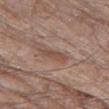Q: Was this lesion biopsied?
A: total-body-photography surveillance lesion; no biopsy
Q: Patient demographics?
A: male, aged 78–82
Q: What is the anatomic site?
A: the leg
Q: What kind of image is this?
A: ~15 mm tile from a whole-body skin photo